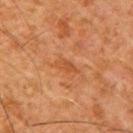<record>
<biopsy_status>not biopsied; imaged during a skin examination</biopsy_status>
<lighting>cross-polarized</lighting>
<site>right upper arm</site>
<automated_metrics>
  <area_mm2_approx>3.0</area_mm2_approx>
  <eccentricity>0.85</eccentricity>
  <shape_asymmetry>0.35</shape_asymmetry>
  <cielab_L>41</cielab_L>
  <cielab_a>23</cielab_a>
  <cielab_b>34</cielab_b>
  <vs_skin_darker_L>6.0</vs_skin_darker_L>
  <vs_skin_contrast_norm>5.5</vs_skin_contrast_norm>
  <border_irregularity_0_10>3.5</border_irregularity_0_10>
  <color_variation_0_10>0.5</color_variation_0_10>
  <peripheral_color_asymmetry>0.0</peripheral_color_asymmetry>
</automated_metrics>
<patient>
  <sex>male</sex>
  <age_approx>65</age_approx>
</patient>
<image>
  <source>total-body photography crop</source>
  <field_of_view_mm>15</field_of_view_mm>
</image>
</record>